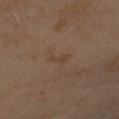No biopsy was performed on this lesion — it was imaged during a full skin examination and was not determined to be concerning. On the left upper arm. Measured at roughly 2.5 mm in maximum diameter. A female patient aged around 60. This image is a 15 mm lesion crop taken from a total-body photograph.